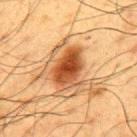| field | value |
|---|---|
| patient | male, in their 60s |
| illumination | cross-polarized |
| automated metrics | an automated nevus-likeness rating near 95 out of 100 and lesion-presence confidence of about 100/100 |
| site | the upper back |
| imaging modality | ~15 mm tile from a whole-body skin photo |